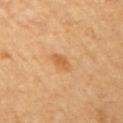workup — total-body-photography surveillance lesion; no biopsy
body site — the right upper arm
lesion size — ~2.5 mm (longest diameter)
image source — 15 mm crop, total-body photography
lighting — cross-polarized
patient — female, aged around 60